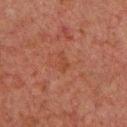{
  "biopsy_status": "not biopsied; imaged during a skin examination",
  "site": "front of the torso",
  "lesion_size": {
    "long_diameter_mm_approx": 2.5
  },
  "image": {
    "source": "total-body photography crop",
    "field_of_view_mm": 15
  },
  "patient": {
    "sex": "male",
    "age_approx": 60
  }
}